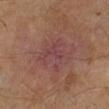biopsy status — no biopsy performed (imaged during a skin exam) | site — the left lower leg | illumination — cross-polarized | image — 15 mm crop, total-body photography | image-analysis metrics — an area of roughly 14 mm²; a mean CIELAB color near L≈42 a*≈22 b*≈20, about 5 CIELAB-L* units darker than the surrounding skin, and a normalized border contrast of about 5.5; a within-lesion color-variation index near 4/10 and peripheral color asymmetry of about 1.5; a lesion-detection confidence of about 100/100 | lesion diameter — ≈4.5 mm | subject — male, aged 53 to 57.